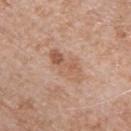The lesion was tiled from a total-body skin photograph and was not biopsied.
A male subject, in their mid- to late 50s.
This is a white-light tile.
The recorded lesion diameter is about 5 mm.
Located on the chest.
Cropped from a whole-body photographic skin survey; the tile spans about 15 mm.
Automated tile analysis of the lesion measured a lesion area of about 10 mm², a shape eccentricity near 0.9, and two-axis asymmetry of about 0.25. The software also gave an average lesion color of about L≈58 a*≈20 b*≈31 (CIELAB), about 8 CIELAB-L* units darker than the surrounding skin, and a lesion-to-skin contrast of about 5.5 (normalized; higher = more distinct). The analysis additionally found a border-irregularity index near 3/10 and a within-lesion color-variation index near 6.5/10. The software also gave a nevus-likeness score of about 0/100 and lesion-presence confidence of about 100/100.